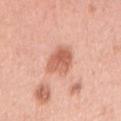Assessment:
The lesion was photographed on a routine skin check and not biopsied; there is no pathology result.
Acquisition and patient details:
From the right upper arm. A 15 mm close-up extracted from a 3D total-body photography capture. A female patient about 60 years old. Imaged with white-light lighting. The total-body-photography lesion software estimated a shape eccentricity near 0.35 and a symmetry-axis asymmetry near 0.2. The analysis additionally found a lesion-detection confidence of about 100/100.